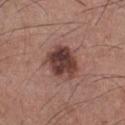image — total-body-photography crop, ~15 mm field of view
site — the chest
subject — male, aged 38–42